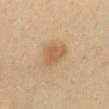The lesion was tiled from a total-body skin photograph and was not biopsied. A female subject aged 38–42. An algorithmic analysis of the crop reported a border-irregularity rating of about 3/10, a color-variation rating of about 3.5/10, and radial color variation of about 1.5. The software also gave a lesion-detection confidence of about 100/100. A 15 mm crop from a total-body photograph taken for skin-cancer surveillance. Located on the mid back. Approximately 3 mm at its widest.